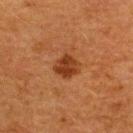Assessment:
Captured during whole-body skin photography for melanoma surveillance; the lesion was not biopsied.
Context:
The tile uses cross-polarized illumination. A female subject aged 38–42. Located on the upper back. A close-up tile cropped from a whole-body skin photograph, about 15 mm across.